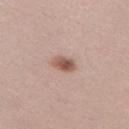The lesion is located on the left thigh.
About 3 mm across.
A 15 mm close-up extracted from a 3D total-body photography capture.
The lesion-visualizer software estimated a footprint of about 4.5 mm². The analysis additionally found a lesion color around L≈55 a*≈19 b*≈26 in CIELAB and a normalized lesion–skin contrast near 9.
The patient is a female aged 38–42.